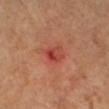{
  "patient": {
    "sex": "female",
    "age_approx": 55
  },
  "lighting": "cross-polarized",
  "image": {
    "source": "total-body photography crop",
    "field_of_view_mm": 15
  },
  "site": "right lower leg"
}